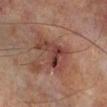{
  "biopsy_status": "not biopsied; imaged during a skin examination",
  "patient": {
    "sex": "male",
    "age_approx": 65
  },
  "site": "left lower leg",
  "automated_metrics": {
    "eccentricity": 0.8,
    "shape_asymmetry": 0.5,
    "cielab_L": 31,
    "cielab_a": 19,
    "cielab_b": 20,
    "vs_skin_darker_L": 8.0,
    "vs_skin_contrast_norm": 8.0,
    "border_irregularity_0_10": 5.5,
    "color_variation_0_10": 6.5,
    "peripheral_color_asymmetry": 2.5,
    "nevus_likeness_0_100": 5,
    "lesion_detection_confidence_0_100": 100
  },
  "image": {
    "source": "total-body photography crop",
    "field_of_view_mm": 15
  },
  "lesion_size": {
    "long_diameter_mm_approx": 5.0
  }
}